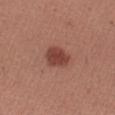notes: imaged on a skin check; not biopsied | illumination: white-light illumination | location: the right upper arm | image source: ~15 mm crop, total-body skin-cancer survey | patient: male, in their mid-30s | lesion diameter: ≈3 mm | automated metrics: a lesion color around L≈43 a*≈26 b*≈26 in CIELAB, a lesion–skin lightness drop of about 12, and a normalized lesion–skin contrast near 9; a classifier nevus-likeness of about 100/100 and lesion-presence confidence of about 100/100.